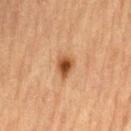No biopsy was performed on this lesion — it was imaged during a full skin examination and was not determined to be concerning. The lesion-visualizer software estimated an area of roughly 5 mm² and an outline eccentricity of about 0.6 (0 = round, 1 = elongated). And it measured an average lesion color of about L≈46 a*≈21 b*≈34 (CIELAB), about 13 CIELAB-L* units darker than the surrounding skin, and a normalized lesion–skin contrast near 9.5. And it measured lesion-presence confidence of about 100/100. A male patient, approximately 85 years of age or older. The lesion is on the lower back. This is a cross-polarized tile. A lesion tile, about 15 mm wide, cut from a 3D total-body photograph. The lesion's longest dimension is about 3 mm.